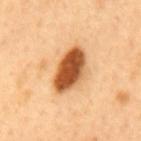Imaged during a routine full-body skin examination; the lesion was not biopsied and no histopathology is available. A male patient aged around 50. A lesion tile, about 15 mm wide, cut from a 3D total-body photograph. The lesion is on the mid back. Captured under cross-polarized illumination.